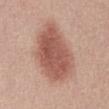biopsy_status: not biopsied; imaged during a skin examination
patient:
  sex: male
  age_approx: 40
lesion_size:
  long_diameter_mm_approx: 7.5
site: abdomen
image:
  source: total-body photography crop
  field_of_view_mm: 15